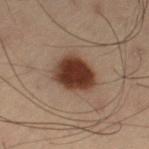Captured during whole-body skin photography for melanoma surveillance; the lesion was not biopsied.